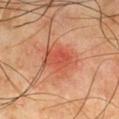– follow-up — total-body-photography surveillance lesion; no biopsy
– acquisition — ~15 mm tile from a whole-body skin photo
– image-analysis metrics — a lesion area of about 11 mm², an eccentricity of roughly 0.85, and two-axis asymmetry of about 0.2; border irregularity of about 2.5 on a 0–10 scale, a within-lesion color-variation index near 3.5/10, and peripheral color asymmetry of about 1; an automated nevus-likeness rating near 90 out of 100 and lesion-presence confidence of about 100/100
– location — the chest
– illumination — cross-polarized
– lesion diameter — ~5 mm (longest diameter)
– subject — male, aged approximately 65